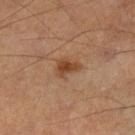biopsy status = catalogued during a skin exam; not biopsied | subject = male, aged 63–67 | body site = the right lower leg | imaging modality = 15 mm crop, total-body photography.